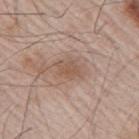The lesion's longest dimension is about 3 mm.
Located on the right upper arm.
The tile uses white-light illumination.
A male patient aged 63–67.
A 15 mm close-up extracted from a 3D total-body photography capture.